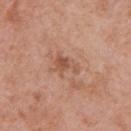workup: catalogued during a skin exam; not biopsied | TBP lesion metrics: a lesion area of about 5 mm², an outline eccentricity of about 0.7 (0 = round, 1 = elongated), and a symmetry-axis asymmetry near 0.4 | illumination: white-light illumination | site: the chest | lesion diameter: about 3 mm | image: ~15 mm crop, total-body skin-cancer survey | patient: male, roughly 70 years of age.